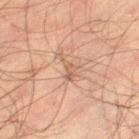• follow-up · imaged on a skin check; not biopsied
• diameter · about 3 mm
• acquisition · 15 mm crop, total-body photography
• tile lighting · cross-polarized illumination
• site · the left thigh
• patient · male, about 50 years old